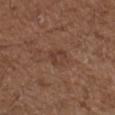  biopsy_status: not biopsied; imaged during a skin examination
  site: arm
  automated_metrics:
    cielab_L: 39
    cielab_a: 19
    cielab_b: 26
    vs_skin_darker_L: 6.0
    vs_skin_contrast_norm: 5.5
  lighting: white-light
  patient:
    sex: male
    age_approx: 50
  image:
    source: total-body photography crop
    field_of_view_mm: 15
  lesion_size:
    long_diameter_mm_approx: 2.5